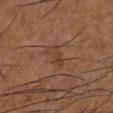Case summary:
- workup: imaged on a skin check; not biopsied
- anatomic site: the left lower leg
- acquisition: ~15 mm tile from a whole-body skin photo
- patient: male, aged approximately 65
- lighting: cross-polarized
- size: about 3 mm
- automated metrics: a lesion area of about 4 mm², an outline eccentricity of about 0.9 (0 = round, 1 = elongated), and two-axis asymmetry of about 0.3; a border-irregularity index near 3.5/10, internal color variation of about 1 on a 0–10 scale, and a peripheral color-asymmetry measure near 0; a classifier nevus-likeness of about 0/100 and a lesion-detection confidence of about 100/100